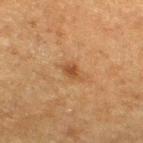The lesion was tiled from a total-body skin photograph and was not biopsied.
The tile uses cross-polarized illumination.
The lesion is located on the right thigh.
Cropped from a whole-body photographic skin survey; the tile spans about 15 mm.
The lesion-visualizer software estimated a mean CIELAB color near L≈40 a*≈19 b*≈32, a lesion–skin lightness drop of about 8, and a lesion-to-skin contrast of about 7 (normalized; higher = more distinct).
A male subject in their mid- to late 70s.